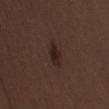Part of a total-body skin-imaging series; this lesion was reviewed on a skin check and was not flagged for biopsy.
A female patient, aged 48–52.
A roughly 15 mm field-of-view crop from a total-body skin photograph.
Captured under white-light illumination.
On the right thigh.